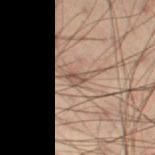Impression: The lesion was tiled from a total-body skin photograph and was not biopsied. Clinical summary: The lesion is located on the left thigh. Imaged with cross-polarized lighting. Cropped from a whole-body photographic skin survey; the tile spans about 15 mm. A male patient, aged 53 to 57. About 3.5 mm across.